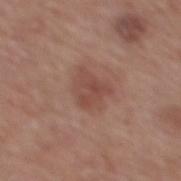biopsy status: total-body-photography surveillance lesion; no biopsy | body site: the back | patient: male, about 75 years old | lighting: white-light illumination | TBP lesion metrics: a mean CIELAB color near L≈47 a*≈22 b*≈25, about 8 CIELAB-L* units darker than the surrounding skin, and a lesion-to-skin contrast of about 6 (normalized; higher = more distinct); a border-irregularity index near 3/10, internal color variation of about 3.5 on a 0–10 scale, and a peripheral color-asymmetry measure near 1; an automated nevus-likeness rating near 35 out of 100 and a detector confidence of about 100 out of 100 that the crop contains a lesion | lesion size: ≈3.5 mm | acquisition: ~15 mm crop, total-body skin-cancer survey.